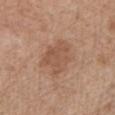follow-up=no biopsy performed (imaged during a skin exam); automated metrics=a nevus-likeness score of about 5/100 and lesion-presence confidence of about 100/100; anatomic site=the chest; acquisition=~15 mm tile from a whole-body skin photo; patient=female, about 70 years old.